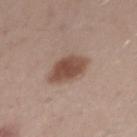Clinical impression:
The lesion was photographed on a routine skin check and not biopsied; there is no pathology result.
Background:
A 15 mm close-up tile from a total-body photography series done for melanoma screening. The subject is a male roughly 30 years of age. This is a white-light tile. The recorded lesion diameter is about 4.5 mm. On the right upper arm.